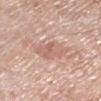Impression:
Imaged during a routine full-body skin examination; the lesion was not biopsied and no histopathology is available.
Background:
The lesion's longest dimension is about 4 mm. On the left lower leg. A roughly 15 mm field-of-view crop from a total-body skin photograph. Captured under white-light illumination. The patient is a male in their 80s.